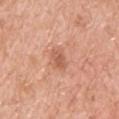An algorithmic analysis of the crop reported a color-variation rating of about 2/10 and peripheral color asymmetry of about 0.5.
A 15 mm crop from a total-body photograph taken for skin-cancer surveillance.
This is a white-light tile.
The lesion is on the upper back.
The patient is a male aged around 55.
Measured at roughly 2.5 mm in maximum diameter.